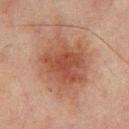workup: imaged on a skin check; not biopsied | location: the back | subject: male, aged 63 to 67 | acquisition: total-body-photography crop, ~15 mm field of view.